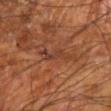Case summary:
* workup — imaged on a skin check; not biopsied
* lesion size — ~4 mm (longest diameter)
* image source — ~15 mm crop, total-body skin-cancer survey
* automated metrics — a border-irregularity index near 6/10, internal color variation of about 3 on a 0–10 scale, and a peripheral color-asymmetry measure near 0.5; a detector confidence of about 85 out of 100 that the crop contains a lesion
* tile lighting — cross-polarized
* anatomic site — the left forearm
* subject — male, aged 68–72